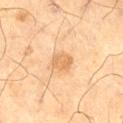Impression:
Part of a total-body skin-imaging series; this lesion was reviewed on a skin check and was not flagged for biopsy.
Image and clinical context:
This is a cross-polarized tile. The total-body-photography lesion software estimated a footprint of about 5 mm², an eccentricity of roughly 0.7, and two-axis asymmetry of about 0.2. The software also gave an average lesion color of about L≈57 a*≈18 b*≈35 (CIELAB), roughly 8 lightness units darker than nearby skin, and a normalized lesion–skin contrast near 6. It also reported a nevus-likeness score of about 10/100 and lesion-presence confidence of about 100/100. Cropped from a total-body skin-imaging series; the visible field is about 15 mm. The patient is a male aged approximately 70. The lesion is on the right thigh. The lesion's longest dimension is about 2.5 mm.